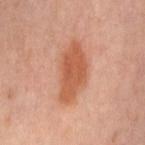Impression: This lesion was catalogued during total-body skin photography and was not selected for biopsy.